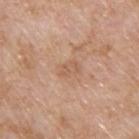Imaged with white-light lighting. The recorded lesion diameter is about 2.5 mm. Automated image analysis of the tile measured an area of roughly 4 mm², an outline eccentricity of about 0.75 (0 = round, 1 = elongated), and two-axis asymmetry of about 0.3. The analysis additionally found a border-irregularity index near 3/10, a within-lesion color-variation index near 2/10, and peripheral color asymmetry of about 0.5. And it measured a nevus-likeness score of about 0/100 and a detector confidence of about 100 out of 100 that the crop contains a lesion. A male subject, aged 58 to 62. Cropped from a whole-body photographic skin survey; the tile spans about 15 mm. Located on the upper back.